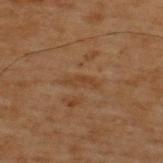biopsy status — imaged on a skin check; not biopsied.